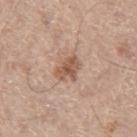Part of a total-body skin-imaging series; this lesion was reviewed on a skin check and was not flagged for biopsy.
Imaged with white-light lighting.
A male patient aged 63 to 67.
A roughly 15 mm field-of-view crop from a total-body skin photograph.
Longest diameter approximately 3 mm.
The lesion is on the right thigh.
The lesion-visualizer software estimated a shape-asymmetry score of about 0.25 (0 = symmetric). The analysis additionally found a border-irregularity rating of about 2.5/10 and peripheral color asymmetry of about 1.